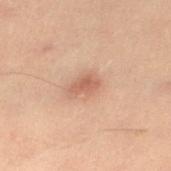{"biopsy_status": "not biopsied; imaged during a skin examination", "lighting": "cross-polarized", "automated_metrics": {"cielab_L": 47, "cielab_a": 19, "cielab_b": 26, "vs_skin_darker_L": 9.0, "vs_skin_contrast_norm": 6.5, "peripheral_color_asymmetry": 0.5}, "patient": {"sex": "female", "age_approx": 30}, "lesion_size": {"long_diameter_mm_approx": 3.0}, "image": {"source": "total-body photography crop", "field_of_view_mm": 15}, "site": "left lower leg"}